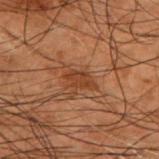workup=no biopsy performed (imaged during a skin exam) | body site=the upper back | lighting=cross-polarized illumination | patient=male, aged around 50 | image=~15 mm crop, total-body skin-cancer survey.